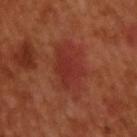follow-up: catalogued during a skin exam; not biopsied | image source: total-body-photography crop, ~15 mm field of view | anatomic site: the upper back | TBP lesion metrics: a border-irregularity index near 3.5/10, a within-lesion color-variation index near 2.5/10, and a peripheral color-asymmetry measure near 0.5; an automated nevus-likeness rating near 40 out of 100 and a lesion-detection confidence of about 100/100 | subject: male, aged around 50 | lighting: cross-polarized | lesion diameter: ~6.5 mm (longest diameter).